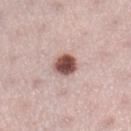Findings:
– notes — catalogued during a skin exam; not biopsied
– lesion diameter — ≈3 mm
– image-analysis metrics — a lesion area of about 6.5 mm² and an outline eccentricity of about 0.55 (0 = round, 1 = elongated); an automated nevus-likeness rating near 100 out of 100
– illumination — white-light illumination
– imaging modality — ~15 mm tile from a whole-body skin photo
– body site — the right lower leg
– patient — female, aged 33 to 37